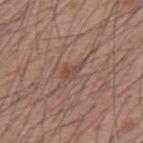biopsy status: no biopsy performed (imaged during a skin exam)
image: total-body-photography crop, ~15 mm field of view
patient: male, aged 58 to 62
body site: the upper back
TBP lesion metrics: a footprint of about 3 mm², a shape eccentricity near 0.85, and a symmetry-axis asymmetry near 0.4; a mean CIELAB color near L≈47 a*≈19 b*≈26, about 7 CIELAB-L* units darker than the surrounding skin, and a lesion-to-skin contrast of about 5.5 (normalized; higher = more distinct); a border-irregularity index near 4/10, a color-variation rating of about 1.5/10, and peripheral color asymmetry of about 0.5; a nevus-likeness score of about 0/100 and a detector confidence of about 90 out of 100 that the crop contains a lesion
size: about 3 mm